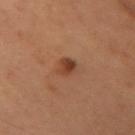* workup · imaged on a skin check; not biopsied
* lighting · cross-polarized
* image source · total-body-photography crop, ~15 mm field of view
* image-analysis metrics · an outline eccentricity of about 0.55 (0 = round, 1 = elongated) and a shape-asymmetry score of about 0.25 (0 = symmetric); a mean CIELAB color near L≈34 a*≈19 b*≈27, roughly 8 lightness units darker than nearby skin, and a normalized border contrast of about 8; border irregularity of about 2 on a 0–10 scale, a color-variation rating of about 4/10, and peripheral color asymmetry of about 1.5
* anatomic site · the front of the torso
* lesion size · ~2.5 mm (longest diameter)
* patient · female, approximately 55 years of age